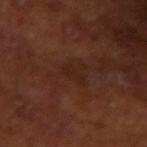follow-up = total-body-photography surveillance lesion; no biopsy.